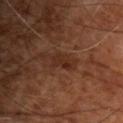Case summary:
– workup — total-body-photography surveillance lesion; no biopsy
– anatomic site — the chest
– automated metrics — internal color variation of about 0.5 on a 0–10 scale; a classifier nevus-likeness of about 0/100 and a detector confidence of about 100 out of 100 that the crop contains a lesion
– diameter — ≈3 mm
– image source — ~15 mm tile from a whole-body skin photo
– patient — male, aged 53–57
– illumination — cross-polarized illumination